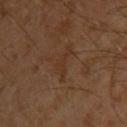workup — imaged on a skin check; not biopsied
site — the upper back
patient — male, aged 28–32
size — about 4 mm
imaging modality — ~15 mm crop, total-body skin-cancer survey
illumination — cross-polarized